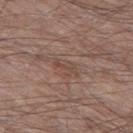{
  "biopsy_status": "not biopsied; imaged during a skin examination",
  "site": "left thigh",
  "lesion_size": {
    "long_diameter_mm_approx": 3.0
  },
  "image": {
    "source": "total-body photography crop",
    "field_of_view_mm": 15
  },
  "patient": {
    "sex": "male",
    "age_approx": 55
  }
}